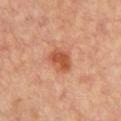follow-up — catalogued during a skin exam; not biopsied | site — the chest | subject — female, about 45 years old | tile lighting — cross-polarized illumination | image source — total-body-photography crop, ~15 mm field of view.